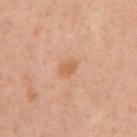Captured during whole-body skin photography for melanoma surveillance; the lesion was not biopsied.